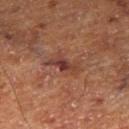lesion diameter: ≈4.5 mm; patient: male, approximately 75 years of age; acquisition: ~15 mm tile from a whole-body skin photo; illumination: cross-polarized illumination; anatomic site: the right thigh.